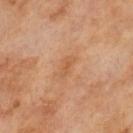No biopsy was performed on this lesion — it was imaged during a full skin examination and was not determined to be concerning. Automated tile analysis of the lesion measured a lesion area of about 3 mm² and a shape-asymmetry score of about 0.25 (0 = symmetric). The analysis additionally found an average lesion color of about L≈55 a*≈23 b*≈36 (CIELAB), about 6 CIELAB-L* units darker than the surrounding skin, and a normalized lesion–skin contrast near 5. And it measured a classifier nevus-likeness of about 0/100 and a detector confidence of about 100 out of 100 that the crop contains a lesion. A 15 mm close-up extracted from a 3D total-body photography capture. A male subject, approximately 65 years of age.